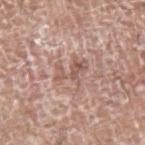<record>
  <biopsy_status>not biopsied; imaged during a skin examination</biopsy_status>
  <site>left upper arm</site>
  <lighting>white-light</lighting>
  <lesion_size>
    <long_diameter_mm_approx>4.5</long_diameter_mm_approx>
  </lesion_size>
  <patient>
    <sex>male</sex>
    <age_approx>75</age_approx>
  </patient>
  <image>
    <source>total-body photography crop</source>
    <field_of_view_mm>15</field_of_view_mm>
  </image>
</record>